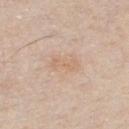workup — imaged on a skin check; not biopsied
patient — male, aged around 30
tile lighting — white-light illumination
lesion diameter — ~3 mm (longest diameter)
TBP lesion metrics — roughly 6 lightness units darker than nearby skin; a classifier nevus-likeness of about 0/100 and a lesion-detection confidence of about 100/100
imaging modality — 15 mm crop, total-body photography
anatomic site — the chest